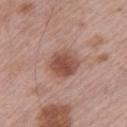follow-up — no biopsy performed (imaged during a skin exam)
lighting — white-light illumination
image source — 15 mm crop, total-body photography
lesion diameter — about 4 mm
anatomic site — the arm
TBP lesion metrics — an area of roughly 10 mm² and an outline eccentricity of about 0.55 (0 = round, 1 = elongated); a within-lesion color-variation index near 4/10 and radial color variation of about 1; an automated nevus-likeness rating near 90 out of 100 and lesion-presence confidence of about 100/100
subject — male, in their mid- to late 60s